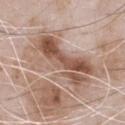This lesion was catalogued during total-body skin photography and was not selected for biopsy. On the front of the torso. Automated tile analysis of the lesion measured a mean CIELAB color near L≈57 a*≈17 b*≈27 and a lesion-to-skin contrast of about 8 (normalized; higher = more distinct). The analysis additionally found a nevus-likeness score of about 0/100 and a detector confidence of about 90 out of 100 that the crop contains a lesion. A male subject, aged approximately 50. A 15 mm crop from a total-body photograph taken for skin-cancer surveillance. This is a white-light tile.